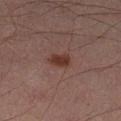{"lighting": "cross-polarized", "patient": {"sex": "male", "age_approx": 30}, "site": "left lower leg", "image": {"source": "total-body photography crop", "field_of_view_mm": 15}, "lesion_size": {"long_diameter_mm_approx": 2.5}, "automated_metrics": {"cielab_L": 28, "cielab_a": 17, "cielab_b": 20, "vs_skin_darker_L": 8.0, "vs_skin_contrast_norm": 9.0, "border_irregularity_0_10": 2.5, "color_variation_0_10": 2.0, "peripheral_color_asymmetry": 0.5}}